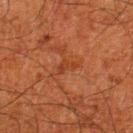Q: Was this lesion biopsied?
A: catalogued during a skin exam; not biopsied
Q: Who is the patient?
A: male, roughly 80 years of age
Q: What is the lesion's diameter?
A: ≈2.5 mm
Q: How was this image acquired?
A: ~15 mm crop, total-body skin-cancer survey
Q: Lesion location?
A: the leg